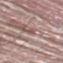Part of a total-body skin-imaging series; this lesion was reviewed on a skin check and was not flagged for biopsy. The lesion-visualizer software estimated an average lesion color of about L≈52 a*≈19 b*≈22 (CIELAB), about 8 CIELAB-L* units darker than the surrounding skin, and a normalized border contrast of about 6. The analysis additionally found a within-lesion color-variation index near 0/10 and radial color variation of about 0. The software also gave an automated nevus-likeness rating near 0 out of 100 and lesion-presence confidence of about 55/100. Located on the mid back. Captured under white-light illumination. Longest diameter approximately 2.5 mm. A male patient aged 38 to 42. A lesion tile, about 15 mm wide, cut from a 3D total-body photograph.